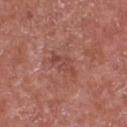* notes: total-body-photography surveillance lesion; no biopsy
* subject: male, aged approximately 65
* automated lesion analysis: a border-irregularity index near 8/10, a within-lesion color-variation index near 0/10, and radial color variation of about 0
* illumination: white-light
* lesion size: ≈3.5 mm
* imaging modality: 15 mm crop, total-body photography
* location: the chest